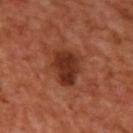Assessment:
Recorded during total-body skin imaging; not selected for excision or biopsy.
Background:
The tile uses cross-polarized illumination. Longest diameter approximately 4 mm. A male patient, aged around 50. This image is a 15 mm lesion crop taken from a total-body photograph. On the upper back.